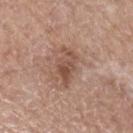No biopsy was performed on this lesion — it was imaged during a full skin examination and was not determined to be concerning.
Cropped from a total-body skin-imaging series; the visible field is about 15 mm.
Captured under white-light illumination.
Measured at roughly 4.5 mm in maximum diameter.
From the left forearm.
The lesion-visualizer software estimated an area of roughly 8 mm², an outline eccentricity of about 0.85 (0 = round, 1 = elongated), and a symmetry-axis asymmetry near 0.3. The analysis additionally found a mean CIELAB color near L≈51 a*≈20 b*≈27 and a normalized lesion–skin contrast near 7. And it measured a border-irregularity rating of about 4/10 and a peripheral color-asymmetry measure near 2. The analysis additionally found a classifier nevus-likeness of about 15/100 and lesion-presence confidence of about 100/100.
A male subject aged 63 to 67.